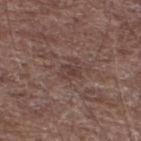workup: imaged on a skin check; not biopsied | site: the left lower leg | lesion size: about 2.5 mm | image: ~15 mm tile from a whole-body skin photo | tile lighting: white-light illumination | patient: male, aged approximately 70.